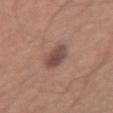Clinical impression: Recorded during total-body skin imaging; not selected for excision or biopsy. Clinical summary: An algorithmic analysis of the crop reported roughly 11 lightness units darker than nearby skin and a lesion-to-skin contrast of about 9 (normalized; higher = more distinct). The analysis additionally found border irregularity of about 2 on a 0–10 scale, a color-variation rating of about 3/10, and radial color variation of about 1. And it measured an automated nevus-likeness rating near 50 out of 100 and a detector confidence of about 100 out of 100 that the crop contains a lesion. A male patient approximately 25 years of age. Measured at roughly 3.5 mm in maximum diameter. The lesion is on the right upper arm. A lesion tile, about 15 mm wide, cut from a 3D total-body photograph.